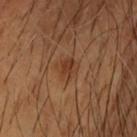| feature | finding |
|---|---|
| biopsy status | total-body-photography surveillance lesion; no biopsy |
| lighting | cross-polarized |
| body site | the head or neck |
| subject | male, aged around 45 |
| acquisition | 15 mm crop, total-body photography |